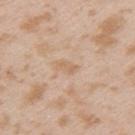The lesion was tiled from a total-body skin photograph and was not biopsied. About 2.5 mm across. Automated tile analysis of the lesion measured a lesion area of about 3 mm² and an outline eccentricity of about 0.85 (0 = round, 1 = elongated). And it measured an automated nevus-likeness rating near 0 out of 100 and a lesion-detection confidence of about 100/100. On the left upper arm. A female patient roughly 25 years of age. Imaged with white-light lighting. A lesion tile, about 15 mm wide, cut from a 3D total-body photograph.